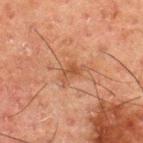<case>
<biopsy_status>not biopsied; imaged during a skin examination</biopsy_status>
<lesion_size>
  <long_diameter_mm_approx>3.0</long_diameter_mm_approx>
</lesion_size>
<site>upper back</site>
<lighting>cross-polarized</lighting>
<patient>
  <sex>male</sex>
  <age_approx>50</age_approx>
</patient>
<image>
  <source>total-body photography crop</source>
  <field_of_view_mm>15</field_of_view_mm>
</image>
</case>